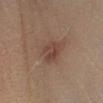Imaged during a routine full-body skin examination; the lesion was not biopsied and no histopathology is available. The subject is a female in their mid- to late 50s. A close-up tile cropped from a whole-body skin photograph, about 15 mm across. The recorded lesion diameter is about 3 mm. On the right lower leg. This is a cross-polarized tile. An algorithmic analysis of the crop reported a mean CIELAB color near L≈35 a*≈16 b*≈21, roughly 6 lightness units darker than nearby skin, and a normalized lesion–skin contrast near 6. The software also gave a color-variation rating of about 3/10 and peripheral color asymmetry of about 1.